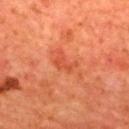Impression: This lesion was catalogued during total-body skin photography and was not selected for biopsy. Image and clinical context: A male subject aged 63–67. The lesion is on the mid back. Longest diameter approximately 3 mm. Imaged with cross-polarized lighting. Automated tile analysis of the lesion measured two-axis asymmetry of about 0.5. And it measured a lesion-to-skin contrast of about 5 (normalized; higher = more distinct). The software also gave lesion-presence confidence of about 100/100. A roughly 15 mm field-of-view crop from a total-body skin photograph.